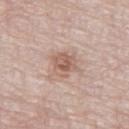  biopsy_status: not biopsied; imaged during a skin examination
  patient:
    sex: male
    age_approx: 80
  lighting: white-light
  image:
    source: total-body photography crop
    field_of_view_mm: 15
  site: left thigh